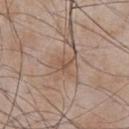follow-up = no biopsy performed (imaged during a skin exam) | anatomic site = the chest | image-analysis metrics = an area of roughly 4 mm² and a symmetry-axis asymmetry near 0.4; an automated nevus-likeness rating near 5 out of 100 and lesion-presence confidence of about 85/100 | size = ≈3 mm | acquisition = 15 mm crop, total-body photography | subject = male, approximately 50 years of age | lighting = white-light.